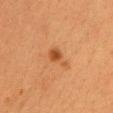The lesion was photographed on a routine skin check and not biopsied; there is no pathology result. Cropped from a total-body skin-imaging series; the visible field is about 15 mm. Captured under cross-polarized illumination. The lesion is on the chest. The patient is a female aged around 55. Longest diameter approximately 3 mm.